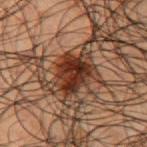Assessment: The lesion was tiled from a total-body skin photograph and was not biopsied. Context: A close-up tile cropped from a whole-body skin photograph, about 15 mm across. Automated image analysis of the tile measured an area of roughly 10 mm² and a shape-asymmetry score of about 0.3 (0 = symmetric). And it measured an average lesion color of about L≈23 a*≈18 b*≈23 (CIELAB), roughly 13 lightness units darker than nearby skin, and a lesion-to-skin contrast of about 13.5 (normalized; higher = more distinct). The analysis additionally found a border-irregularity rating of about 3.5/10 and peripheral color asymmetry of about 1.5. Located on the left upper arm. The patient is a male approximately 50 years of age.